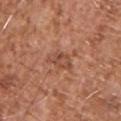Impression: The lesion was photographed on a routine skin check and not biopsied; there is no pathology result. Acquisition and patient details: Captured under white-light illumination. A male patient in their mid- to late 70s. About 4 mm across. Cropped from a total-body skin-imaging series; the visible field is about 15 mm. From the upper back.